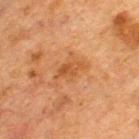Notes:
- follow-up — catalogued during a skin exam; not biopsied
- acquisition — ~15 mm tile from a whole-body skin photo
- tile lighting — cross-polarized
- location — the back
- subject — male, roughly 50 years of age
- diameter — ~3 mm (longest diameter)